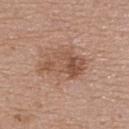notes: imaged on a skin check; not biopsied
lesion size: ≈5.5 mm
acquisition: 15 mm crop, total-body photography
lighting: white-light illumination
anatomic site: the back
patient: female, aged around 60